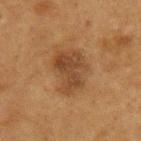notes: total-body-photography surveillance lesion; no biopsy | location: the upper back | image: ~15 mm crop, total-body skin-cancer survey | patient: male, approximately 75 years of age.